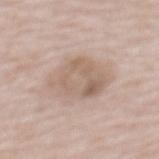patient = female, roughly 65 years of age | image = 15 mm crop, total-body photography | size = ~6 mm (longest diameter) | tile lighting = white-light illumination | anatomic site = the mid back | image-analysis metrics = an automated nevus-likeness rating near 5 out of 100 and lesion-presence confidence of about 100/100.